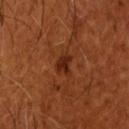The lesion was tiled from a total-body skin photograph and was not biopsied.
A 15 mm crop from a total-body photograph taken for skin-cancer surveillance.
The lesion is located on the head or neck.
A male subject, aged approximately 65.